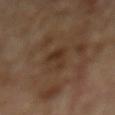Impression:
Captured during whole-body skin photography for melanoma surveillance; the lesion was not biopsied.
Context:
On the mid back. A close-up tile cropped from a whole-body skin photograph, about 15 mm across. Automated image analysis of the tile measured an average lesion color of about L≈31 a*≈15 b*≈27 (CIELAB), roughly 7 lightness units darker than nearby skin, and a normalized border contrast of about 7. The analysis additionally found lesion-presence confidence of about 100/100. The subject is a male aged 68–72. Approximately 3.5 mm at its widest. Captured under cross-polarized illumination.